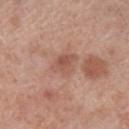| field | value |
|---|---|
| follow-up | catalogued during a skin exam; not biopsied |
| size | ~3.5 mm (longest diameter) |
| body site | the left lower leg |
| image | total-body-photography crop, ~15 mm field of view |
| patient | male, aged 68–72 |
| illumination | white-light |